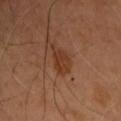Assessment: Recorded during total-body skin imaging; not selected for excision or biopsy. Acquisition and patient details: The patient is a male aged 53 to 57. A close-up tile cropped from a whole-body skin photograph, about 15 mm across. The lesion is located on the chest. Imaged with cross-polarized lighting. Approximately 4 mm at its widest.